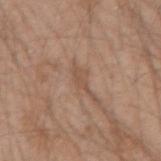biopsy status: imaged on a skin check; not biopsied
automated metrics: border irregularity of about 4.5 on a 0–10 scale, internal color variation of about 0 on a 0–10 scale, and a peripheral color-asymmetry measure near 0; a classifier nevus-likeness of about 0/100
lesion size: ~3 mm (longest diameter)
subject: male, aged around 20
anatomic site: the right upper arm
lighting: white-light
imaging modality: ~15 mm tile from a whole-body skin photo